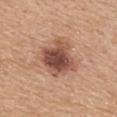biopsy status: imaged on a skin check; not biopsied | site: the upper back | patient: female, in their mid-50s | image: ~15 mm tile from a whole-body skin photo.